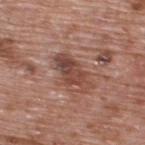Assessment: Imaged during a routine full-body skin examination; the lesion was not biopsied and no histopathology is available. Background: Longest diameter approximately 5 mm. Automated tile analysis of the lesion measured a lesion color around L≈45 a*≈22 b*≈25 in CIELAB, roughly 11 lightness units darker than nearby skin, and a normalized lesion–skin contrast near 8. It also reported a border-irregularity index near 4.5/10, a color-variation rating of about 5/10, and peripheral color asymmetry of about 1.5. And it measured lesion-presence confidence of about 100/100. Located on the upper back. Imaged with white-light lighting. The subject is a male roughly 70 years of age. Cropped from a whole-body photographic skin survey; the tile spans about 15 mm.